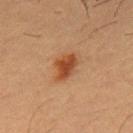Context:
A 15 mm crop from a total-body photograph taken for skin-cancer surveillance. Imaged with cross-polarized lighting. Longest diameter approximately 3.5 mm. A male subject roughly 55 years of age. Automated image analysis of the tile measured an outline eccentricity of about 0.75 (0 = round, 1 = elongated) and a shape-asymmetry score of about 0.3 (0 = symmetric). It also reported an average lesion color of about L≈40 a*≈23 b*≈33 (CIELAB) and a lesion-to-skin contrast of about 9.5 (normalized; higher = more distinct). The lesion is on the front of the torso.